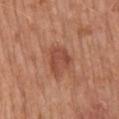On the mid back. A roughly 15 mm field-of-view crop from a total-body skin photograph. A male patient approximately 70 years of age.